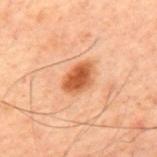Part of a total-body skin-imaging series; this lesion was reviewed on a skin check and was not flagged for biopsy.